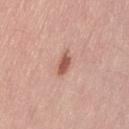biopsy status = total-body-photography surveillance lesion; no biopsy
illumination = white-light illumination
imaging modality = ~15 mm tile from a whole-body skin photo
anatomic site = the left thigh
TBP lesion metrics = a mean CIELAB color near L≈56 a*≈24 b*≈28 and roughly 13 lightness units darker than nearby skin; a border-irregularity rating of about 2/10 and a peripheral color-asymmetry measure near 1; an automated nevus-likeness rating near 85 out of 100 and lesion-presence confidence of about 100/100
diameter = ~3 mm (longest diameter)
patient = male, aged 23 to 27